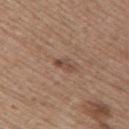Assessment: The lesion was photographed on a routine skin check and not biopsied; there is no pathology result. Image and clinical context: Captured under white-light illumination. On the upper back. Approximately 2.5 mm at its widest. A female patient, about 60 years old. A lesion tile, about 15 mm wide, cut from a 3D total-body photograph. Automated tile analysis of the lesion measured a lesion color around L≈46 a*≈19 b*≈27 in CIELAB.